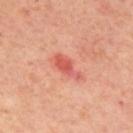Q: Was this lesion biopsied?
A: catalogued during a skin exam; not biopsied